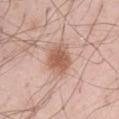Imaged during a routine full-body skin examination; the lesion was not biopsied and no histopathology is available. A 15 mm close-up tile from a total-body photography series done for melanoma screening. Automated tile analysis of the lesion measured a lesion area of about 9.5 mm², a shape eccentricity near 0.55, and a shape-asymmetry score of about 0.2 (0 = symmetric). And it measured a lesion color around L≈59 a*≈22 b*≈29 in CIELAB, about 12 CIELAB-L* units darker than the surrounding skin, and a lesion-to-skin contrast of about 8 (normalized; higher = more distinct). The analysis additionally found a within-lesion color-variation index near 3.5/10 and radial color variation of about 1. And it measured a classifier nevus-likeness of about 95/100 and lesion-presence confidence of about 100/100. A male subject roughly 55 years of age. The lesion's longest dimension is about 4 mm. On the left thigh.